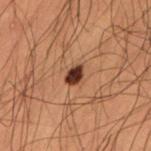The lesion was tiled from a total-body skin photograph and was not biopsied. The total-body-photography lesion software estimated a footprint of about 4 mm², an eccentricity of roughly 0.65, and two-axis asymmetry of about 0.2. The analysis additionally found a border-irregularity rating of about 2/10, a within-lesion color-variation index near 3.5/10, and peripheral color asymmetry of about 1. The analysis additionally found a nevus-likeness score of about 100/100 and a lesion-detection confidence of about 100/100. A 15 mm close-up tile from a total-body photography series done for melanoma screening. A male patient about 50 years old. The tile uses cross-polarized illumination. Located on the left thigh. About 2.5 mm across.